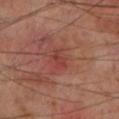Recorded during total-body skin imaging; not selected for excision or biopsy. The subject is a male about 70 years old. A close-up tile cropped from a whole-body skin photograph, about 15 mm across. Automated tile analysis of the lesion measured an area of roughly 3 mm² and a symmetry-axis asymmetry near 0.4. And it measured a border-irregularity rating of about 4.5/10, internal color variation of about 1 on a 0–10 scale, and a peripheral color-asymmetry measure near 0. The lesion's longest dimension is about 2.5 mm. The tile uses cross-polarized illumination. The lesion is located on the left lower leg.